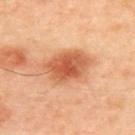The lesion was photographed on a routine skin check and not biopsied; there is no pathology result. The lesion-visualizer software estimated an area of roughly 16 mm². The software also gave a mean CIELAB color near L≈62 a*≈29 b*≈40 and a lesion-to-skin contrast of about 8.5 (normalized; higher = more distinct). The software also gave a border-irregularity rating of about 2/10, a within-lesion color-variation index near 5.5/10, and a peripheral color-asymmetry measure near 1.5. The subject is a female aged 38–42. Approximately 6 mm at its widest. The tile uses cross-polarized illumination. A 15 mm close-up tile from a total-body photography series done for melanoma screening. The lesion is located on the upper back.